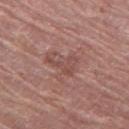Recorded during total-body skin imaging; not selected for excision or biopsy. The lesion-visualizer software estimated a mean CIELAB color near L≈48 a*≈22 b*≈23 and roughly 7 lightness units darker than nearby skin. From the left thigh. A female patient, aged around 80. Captured under white-light illumination. Cropped from a whole-body photographic skin survey; the tile spans about 15 mm.